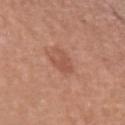This lesion was catalogued during total-body skin photography and was not selected for biopsy. On the left upper arm. A region of skin cropped from a whole-body photographic capture, roughly 15 mm wide. A female patient approximately 50 years of age.